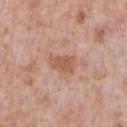biopsy status — total-body-photography surveillance lesion; no biopsy
imaging modality — total-body-photography crop, ~15 mm field of view
location — the chest
subject — male, approximately 60 years of age